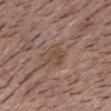Impression:
Part of a total-body skin-imaging series; this lesion was reviewed on a skin check and was not flagged for biopsy.
Background:
Located on the head or neck. The patient is a male aged around 40. The recorded lesion diameter is about 3 mm. A roughly 15 mm field-of-view crop from a total-body skin photograph.